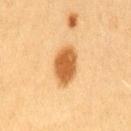workup = total-body-photography surveillance lesion; no biopsy
imaging modality = total-body-photography crop, ~15 mm field of view
subject = female, aged 53–57
lesion diameter = about 4.5 mm
automated metrics = an average lesion color of about L≈52 a*≈22 b*≈40 (CIELAB) and a normalized border contrast of about 10; border irregularity of about 1.5 on a 0–10 scale, a color-variation rating of about 2.5/10, and radial color variation of about 1
illumination = cross-polarized illumination
body site = the back